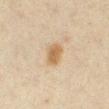follow-up: no biopsy performed (imaged during a skin exam)
patient: female, approximately 45 years of age
diameter: ≈3 mm
site: the right lower leg
tile lighting: cross-polarized
automated lesion analysis: roughly 9 lightness units darker than nearby skin and a lesion-to-skin contrast of about 8 (normalized; higher = more distinct); an automated nevus-likeness rating near 95 out of 100 and a detector confidence of about 100 out of 100 that the crop contains a lesion
image source: 15 mm crop, total-body photography